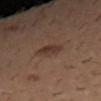Q: Is there a histopathology result?
A: catalogued during a skin exam; not biopsied
Q: How was the tile lit?
A: cross-polarized
Q: How large is the lesion?
A: ~2.5 mm (longest diameter)
Q: Where on the body is the lesion?
A: the right forearm
Q: What kind of image is this?
A: ~15 mm crop, total-body skin-cancer survey
Q: What are the patient's age and sex?
A: male, approximately 30 years of age